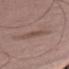Notes:
– biopsy status · total-body-photography surveillance lesion; no biopsy
– anatomic site · the left thigh
– subject · male, aged approximately 75
– tile lighting · white-light illumination
– diameter · about 2.5 mm
– acquisition · 15 mm crop, total-body photography